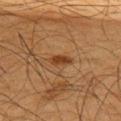follow-up = total-body-photography surveillance lesion; no biopsy | patient = male, about 65 years old | illumination = cross-polarized | image source = 15 mm crop, total-body photography | anatomic site = the upper back | lesion size = ~3 mm (longest diameter).